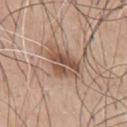The subject is a male in their mid-60s.
Approximately 5 mm at its widest.
The tile uses white-light illumination.
A region of skin cropped from a whole-body photographic capture, roughly 15 mm wide.
From the chest.